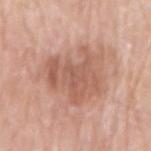{"biopsy_status": "not biopsied; imaged during a skin examination", "site": "arm", "image": {"source": "total-body photography crop", "field_of_view_mm": 15}, "patient": {"sex": "male", "age_approx": 80}}